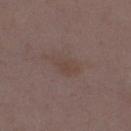Captured during whole-body skin photography for melanoma surveillance; the lesion was not biopsied. The tile uses white-light illumination. Automated image analysis of the tile measured an area of roughly 6 mm², an outline eccentricity of about 0.85 (0 = round, 1 = elongated), and a symmetry-axis asymmetry near 0.25. The analysis additionally found border irregularity of about 3 on a 0–10 scale and internal color variation of about 1.5 on a 0–10 scale. A female subject, in their mid-30s. A 15 mm close-up extracted from a 3D total-body photography capture. Located on the abdomen. The recorded lesion diameter is about 3.5 mm.